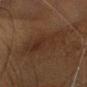* subject — female, approximately 40 years of age
* site — the head or neck
* image source — total-body-photography crop, ~15 mm field of view